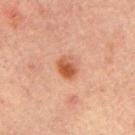biopsy status: no biopsy performed (imaged during a skin exam) | subject: male, about 30 years old | image source: 15 mm crop, total-body photography | location: the right upper arm.